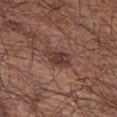The lesion was photographed on a routine skin check and not biopsied; there is no pathology result. The lesion's longest dimension is about 3.5 mm. A close-up tile cropped from a whole-body skin photograph, about 15 mm across. Automated image analysis of the tile measured a mean CIELAB color near L≈37 a*≈19 b*≈23, a lesion–skin lightness drop of about 9, and a normalized border contrast of about 8. A male subject about 65 years old. On the right upper arm.